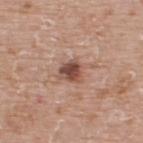<case>
  <biopsy_status>not biopsied; imaged during a skin examination</biopsy_status>
  <automated_metrics>
    <border_irregularity_0_10>2.5</border_irregularity_0_10>
    <color_variation_0_10>5.0</color_variation_0_10>
    <peripheral_color_asymmetry>1.5</peripheral_color_asymmetry>
  </automated_metrics>
  <image>
    <source>total-body photography crop</source>
    <field_of_view_mm>15</field_of_view_mm>
  </image>
  <lesion_size>
    <long_diameter_mm_approx>2.5</long_diameter_mm_approx>
  </lesion_size>
  <patient>
    <sex>male</sex>
    <age_approx>75</age_approx>
  </patient>
  <lighting>white-light</lighting>
  <site>upper back</site>
</case>